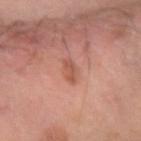Q: Is there a histopathology result?
A: total-body-photography surveillance lesion; no biopsy
Q: Lesion location?
A: the left forearm
Q: What did automated image analysis measure?
A: a footprint of about 3 mm², an outline eccentricity of about 0.9 (0 = round, 1 = elongated), and a shape-asymmetry score of about 0.4 (0 = symmetric); a lesion color around L≈51 a*≈25 b*≈29 in CIELAB and a normalized border contrast of about 6; a border-irregularity index near 4/10 and internal color variation of about 0.5 on a 0–10 scale
Q: What kind of image is this?
A: ~15 mm tile from a whole-body skin photo
Q: What is the lesion's diameter?
A: ~3 mm (longest diameter)
Q: What are the patient's age and sex?
A: male, in their 60s